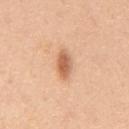biopsy status: imaged on a skin check; not biopsied | tile lighting: white-light | image: ~15 mm crop, total-body skin-cancer survey | subject: male, aged 48–52 | site: the abdomen | diameter: ~3.5 mm (longest diameter) | image-analysis metrics: an eccentricity of roughly 0.85 and two-axis asymmetry of about 0.15; roughly 13 lightness units darker than nearby skin and a normalized lesion–skin contrast near 8; a border-irregularity index near 2/10 and radial color variation of about 1.